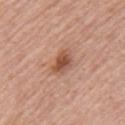Assessment: Recorded during total-body skin imaging; not selected for excision or biopsy. Context: A 15 mm crop from a total-body photograph taken for skin-cancer surveillance. On the left upper arm. The tile uses white-light illumination. The lesion's longest dimension is about 3 mm. A female patient about 65 years old.